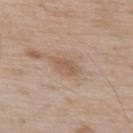notes=imaged on a skin check; not biopsied
lighting=white-light illumination
size=~3 mm (longest diameter)
subject=male, about 55 years old
imaging modality=total-body-photography crop, ~15 mm field of view
TBP lesion metrics=an automated nevus-likeness rating near 0 out of 100 and a detector confidence of about 100 out of 100 that the crop contains a lesion
location=the upper back